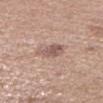No biopsy was performed on this lesion — it was imaged during a full skin examination and was not determined to be concerning.